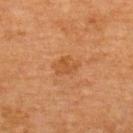| feature | finding |
|---|---|
| notes | total-body-photography surveillance lesion; no biopsy |
| anatomic site | the upper back |
| image-analysis metrics | a lesion-detection confidence of about 100/100 |
| subject | female, in their mid-50s |
| imaging modality | 15 mm crop, total-body photography |
| illumination | cross-polarized illumination |